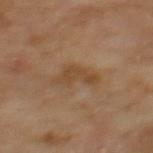The lesion was photographed on a routine skin check and not biopsied; there is no pathology result. Cropped from a whole-body photographic skin survey; the tile spans about 15 mm. The lesion's longest dimension is about 4 mm. Captured under cross-polarized illumination. A male subject aged 68 to 72. Located on the mid back.